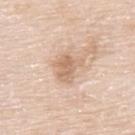Assessment:
This lesion was catalogued during total-body skin photography and was not selected for biopsy.
Acquisition and patient details:
On the upper back. The subject is a male aged approximately 75. This image is a 15 mm lesion crop taken from a total-body photograph. The recorded lesion diameter is about 3.5 mm. Captured under white-light illumination.